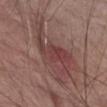Clinical impression:
No biopsy was performed on this lesion — it was imaged during a full skin examination and was not determined to be concerning.
Image and clinical context:
On the chest. A region of skin cropped from a whole-body photographic capture, roughly 15 mm wide. The patient is a male aged approximately 70. Captured under white-light illumination. Automated tile analysis of the lesion measured an average lesion color of about L≈43 a*≈21 b*≈20 (CIELAB), a lesion–skin lightness drop of about 8, and a normalized border contrast of about 6.5. The analysis additionally found a border-irregularity rating of about 7/10 and a within-lesion color-variation index near 6/10. The analysis additionally found an automated nevus-likeness rating near 5 out of 100 and lesion-presence confidence of about 100/100. The lesion's longest dimension is about 10.5 mm.